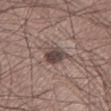Impression: No biopsy was performed on this lesion — it was imaged during a full skin examination and was not determined to be concerning. Image and clinical context: From the left lower leg. The subject is a male aged approximately 35. About 3 mm across. Automated tile analysis of the lesion measured a lesion color around L≈43 a*≈14 b*≈18 in CIELAB and roughly 13 lightness units darker than nearby skin. The software also gave a border-irregularity index near 2/10, internal color variation of about 3.5 on a 0–10 scale, and a peripheral color-asymmetry measure near 1. Imaged with white-light lighting. A close-up tile cropped from a whole-body skin photograph, about 15 mm across.